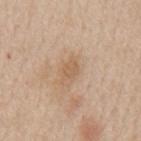Part of a total-body skin-imaging series; this lesion was reviewed on a skin check and was not flagged for biopsy. An algorithmic analysis of the crop reported a color-variation rating of about 1/10 and a peripheral color-asymmetry measure near 0.5. It also reported an automated nevus-likeness rating near 5 out of 100 and a lesion-detection confidence of about 100/100. A male subject, roughly 60 years of age. This is a white-light tile. The lesion is located on the mid back. A 15 mm crop from a total-body photograph taken for skin-cancer surveillance. Approximately 2.5 mm at its widest.